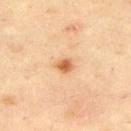{
  "lighting": "cross-polarized",
  "automated_metrics": {
    "eccentricity": 0.6,
    "shape_asymmetry": 0.2,
    "cielab_L": 62,
    "cielab_a": 25,
    "cielab_b": 41,
    "vs_skin_contrast_norm": 9.0,
    "nevus_likeness_0_100": 95
  },
  "lesion_size": {
    "long_diameter_mm_approx": 2.0
  },
  "image": {
    "source": "total-body photography crop",
    "field_of_view_mm": 15
  },
  "patient": {
    "sex": "male",
    "age_approx": 45
  },
  "site": "upper back"
}